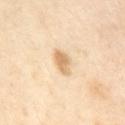Case summary:
• notes: no biopsy performed (imaged during a skin exam)
• anatomic site: the mid back
• patient: female, in their mid- to late 50s
• image: ~15 mm tile from a whole-body skin photo